A male subject, aged 58 to 62 · a 15 mm crop from a total-body photograph taken for skin-cancer surveillance · the lesion is located on the left thigh — 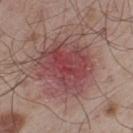Findings:
- diagnosis: a nodular basal cell carcinoma